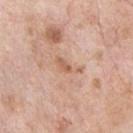Captured during whole-body skin photography for melanoma surveillance; the lesion was not biopsied. The subject is a male aged approximately 70. Cropped from a whole-body photographic skin survey; the tile spans about 15 mm. The lesion is on the front of the torso.